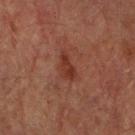subject = male, in their mid- to late 70s; body site = the arm; diameter = ≈3.5 mm; lighting = cross-polarized; acquisition = ~15 mm tile from a whole-body skin photo.